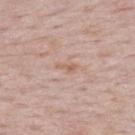| key | value |
|---|---|
| follow-up | catalogued during a skin exam; not biopsied |
| image source | ~15 mm crop, total-body skin-cancer survey |
| lesion diameter | ≈2.5 mm |
| lighting | white-light |
| location | the back |
| patient | female, aged 48–52 |